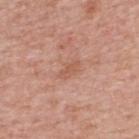The subject is a female approximately 60 years of age.
A lesion tile, about 15 mm wide, cut from a 3D total-body photograph.
An algorithmic analysis of the crop reported a shape eccentricity near 0.85 and a shape-asymmetry score of about 0.35 (0 = symmetric). It also reported a nevus-likeness score of about 0/100 and a detector confidence of about 100 out of 100 that the crop contains a lesion.
Captured under white-light illumination.
The recorded lesion diameter is about 3 mm.
Located on the upper back.